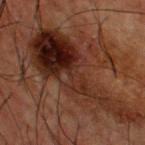Findings:
* biopsy status — catalogued during a skin exam; not biopsied
* anatomic site — the back
* subject — male, roughly 50 years of age
* image — ~15 mm crop, total-body skin-cancer survey